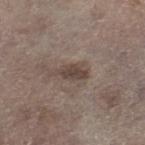Q: Is there a histopathology result?
A: total-body-photography surveillance lesion; no biopsy
Q: What is the imaging modality?
A: ~15 mm tile from a whole-body skin photo
Q: How was the tile lit?
A: white-light illumination
Q: Lesion size?
A: ≈3.5 mm
Q: Where on the body is the lesion?
A: the right lower leg
Q: Who is the patient?
A: male, aged 68–72